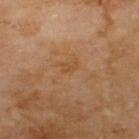location: the upper back | subject: male, aged approximately 70 | lesion size: ≈1.5 mm | image source: ~15 mm crop, total-body skin-cancer survey | lighting: cross-polarized.